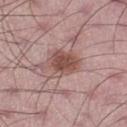workup: no biopsy performed (imaged during a skin exam); anatomic site: the right thigh; image source: ~15 mm crop, total-body skin-cancer survey; patient: male, roughly 50 years of age; lighting: white-light illumination; automated lesion analysis: roughly 12 lightness units darker than nearby skin.